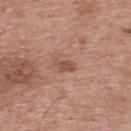Findings:
- follow-up — no biopsy performed (imaged during a skin exam)
- size — ≈2.5 mm
- illumination — white-light
- anatomic site — the upper back
- imaging modality — ~15 mm tile from a whole-body skin photo
- patient — male, roughly 65 years of age